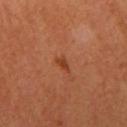acquisition: ~15 mm crop, total-body skin-cancer survey | automated metrics: an area of roughly 2 mm², an outline eccentricity of about 0.8 (0 = round, 1 = elongated), and a symmetry-axis asymmetry near 0.4; a within-lesion color-variation index near 0/10 and radial color variation of about 0 | location: the left arm | illumination: cross-polarized illumination | subject: female, aged 63–67 | lesion size: about 2 mm.